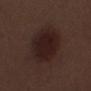The lesion was tiled from a total-body skin photograph and was not biopsied.
From the left lower leg.
A lesion tile, about 15 mm wide, cut from a 3D total-body photograph.
Automated image analysis of the tile measured an average lesion color of about L≈19 a*≈16 b*≈16 (CIELAB). The software also gave a border-irregularity rating of about 1/10 and a within-lesion color-variation index near 3.5/10. The software also gave a nevus-likeness score of about 75/100 and lesion-presence confidence of about 100/100.
A male patient, in their 70s.